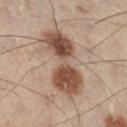Q: Is there a histopathology result?
A: no biopsy performed (imaged during a skin exam)
Q: How was the tile lit?
A: cross-polarized
Q: Where on the body is the lesion?
A: the right lower leg
Q: Automated lesion metrics?
A: an area of roughly 22 mm² and a symmetry-axis asymmetry near 0.25
Q: Who is the patient?
A: male, approximately 55 years of age
Q: How was this image acquired?
A: total-body-photography crop, ~15 mm field of view
Q: What is the lesion's diameter?
A: ~7.5 mm (longest diameter)